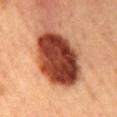Q: Automated lesion metrics?
A: a lesion color around L≈38 a*≈27 b*≈31 in CIELAB, roughly 23 lightness units darker than nearby skin, and a lesion-to-skin contrast of about 16 (normalized; higher = more distinct); lesion-presence confidence of about 100/100
Q: How was the tile lit?
A: cross-polarized illumination
Q: Who is the patient?
A: female, aged 58 to 62
Q: Where on the body is the lesion?
A: the back
Q: What is the lesion's diameter?
A: ≈8 mm
Q: What is the imaging modality?
A: ~15 mm tile from a whole-body skin photo